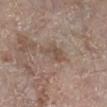Impression:
Imaged during a routine full-body skin examination; the lesion was not biopsied and no histopathology is available.
Acquisition and patient details:
A male subject, roughly 65 years of age. The lesion's longest dimension is about 4 mm. This is a white-light tile. Automated tile analysis of the lesion measured a lesion color around L≈50 a*≈14 b*≈24 in CIELAB and a lesion-to-skin contrast of about 6 (normalized; higher = more distinct). And it measured a border-irregularity rating of about 4/10 and internal color variation of about 2.5 on a 0–10 scale. A 15 mm close-up extracted from a 3D total-body photography capture. From the leg.